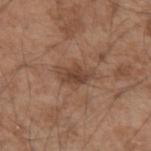notes = no biopsy performed (imaged during a skin exam)
automated lesion analysis = a lesion color around L≈42 a*≈19 b*≈28 in CIELAB, roughly 10 lightness units darker than nearby skin, and a normalized border contrast of about 8; a border-irregularity index near 2.5/10, a within-lesion color-variation index near 2/10, and radial color variation of about 0.5; a classifier nevus-likeness of about 25/100 and a lesion-detection confidence of about 100/100
image source = 15 mm crop, total-body photography
subject = male, aged 53 to 57
lesion size = about 3.5 mm
location = the left upper arm